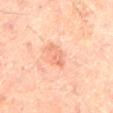Impression:
The lesion was tiled from a total-body skin photograph and was not biopsied.
Context:
A male patient in their mid-60s. The total-body-photography lesion software estimated a footprint of about 4.5 mm², an outline eccentricity of about 0.85 (0 = round, 1 = elongated), and two-axis asymmetry of about 0.3. And it measured a lesion–skin lightness drop of about 9 and a lesion-to-skin contrast of about 5.5 (normalized; higher = more distinct). And it measured border irregularity of about 3 on a 0–10 scale and a within-lesion color-variation index near 3/10. The lesion's longest dimension is about 3.5 mm. A 15 mm crop from a total-body photograph taken for skin-cancer surveillance. On the lower back.